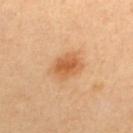This lesion was catalogued during total-body skin photography and was not selected for biopsy.